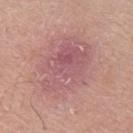| feature | finding |
|---|---|
| follow-up | imaged on a skin check; not biopsied |
| subject | male, in their 60s |
| image | ~15 mm tile from a whole-body skin photo |
| lesion diameter | about 7.5 mm |
| site | the arm |